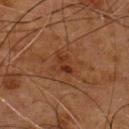Q: Is there a histopathology result?
A: catalogued during a skin exam; not biopsied
Q: What is the imaging modality?
A: ~15 mm tile from a whole-body skin photo
Q: What is the anatomic site?
A: the chest
Q: Automated lesion metrics?
A: an area of roughly 3 mm², an outline eccentricity of about 0.85 (0 = round, 1 = elongated), and a shape-asymmetry score of about 0.6 (0 = symmetric); a lesion color around L≈30 a*≈23 b*≈30 in CIELAB, roughly 7 lightness units darker than nearby skin, and a lesion-to-skin contrast of about 7.5 (normalized; higher = more distinct); a border-irregularity index near 6/10; an automated nevus-likeness rating near 0 out of 100 and a lesion-detection confidence of about 100/100
Q: How large is the lesion?
A: ≈2.5 mm
Q: Illumination type?
A: cross-polarized
Q: Who is the patient?
A: male, about 55 years old